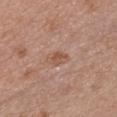No biopsy was performed on this lesion — it was imaged during a full skin examination and was not determined to be concerning. Approximately 2.5 mm at its widest. A female subject approximately 50 years of age. The tile uses white-light illumination. A 15 mm close-up extracted from a 3D total-body photography capture. The lesion is on the chest.